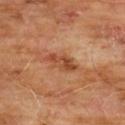Findings:
• workup: imaged on a skin check; not biopsied
• lesion diameter: ~4 mm (longest diameter)
• subject: male, approximately 60 years of age
• image source: total-body-photography crop, ~15 mm field of view
• illumination: cross-polarized
• anatomic site: the front of the torso